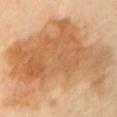Clinical impression:
Captured during whole-body skin photography for melanoma surveillance; the lesion was not biopsied.
Clinical summary:
A male subject about 70 years old. A 15 mm close-up tile from a total-body photography series done for melanoma screening. The lesion is located on the left upper arm. Imaged with cross-polarized lighting. About 13.5 mm across.